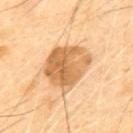{"biopsy_status": "not biopsied; imaged during a skin examination", "site": "mid back", "image": {"source": "total-body photography crop", "field_of_view_mm": 15}, "lesion_size": {"long_diameter_mm_approx": 5.5}, "patient": {"sex": "male", "age_approx": 70}, "lighting": "cross-polarized"}